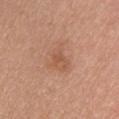Imaged during a routine full-body skin examination; the lesion was not biopsied and no histopathology is available.
An algorithmic analysis of the crop reported a lesion area of about 7 mm² and a symmetry-axis asymmetry near 0.5. The analysis additionally found a lesion color around L≈56 a*≈22 b*≈32 in CIELAB, about 7 CIELAB-L* units darker than the surrounding skin, and a normalized border contrast of about 5.
The lesion is on the back.
This image is a 15 mm lesion crop taken from a total-body photograph.
Captured under white-light illumination.
The subject is a female approximately 40 years of age.